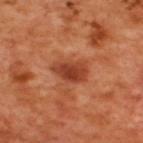Part of a total-body skin-imaging series; this lesion was reviewed on a skin check and was not flagged for biopsy. A male patient, in their 50s. On the upper back. This is a cross-polarized tile. Cropped from a whole-body photographic skin survey; the tile spans about 15 mm. The recorded lesion diameter is about 4 mm.